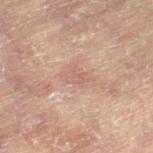Notes:
* workup · imaged on a skin check; not biopsied
* body site · the right leg
* image · 15 mm crop, total-body photography
* patient · female, in their 80s
* lesion diameter · ≈3.5 mm